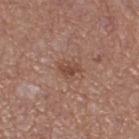Impression:
The lesion was photographed on a routine skin check and not biopsied; there is no pathology result.
Image and clinical context:
A female patient aged 43 to 47. A lesion tile, about 15 mm wide, cut from a 3D total-body photograph. From the right thigh.